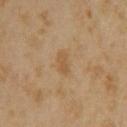<tbp_lesion>
<biopsy_status>not biopsied; imaged during a skin examination</biopsy_status>
<image>
  <source>total-body photography crop</source>
  <field_of_view_mm>15</field_of_view_mm>
</image>
<lesion_size>
  <long_diameter_mm_approx>2.5</long_diameter_mm_approx>
</lesion_size>
<site>head or neck</site>
<lighting>cross-polarized</lighting>
<patient>
  <sex>female</sex>
  <age_approx>35</age_approx>
</patient>
<automated_metrics>
  <cielab_L>51</cielab_L>
  <cielab_a>16</cielab_a>
  <cielab_b>36</cielab_b>
  <vs_skin_contrast_norm>6.0</vs_skin_contrast_norm>
  <lesion_detection_confidence_0_100>100</lesion_detection_confidence_0_100>
</automated_metrics>
</tbp_lesion>